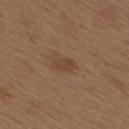The lesion was tiled from a total-body skin photograph and was not biopsied. Approximately 3 mm at its widest. The total-body-photography lesion software estimated an automated nevus-likeness rating near 5 out of 100. The patient is a male in their mid- to late 70s. From the mid back. The tile uses white-light illumination. A lesion tile, about 15 mm wide, cut from a 3D total-body photograph.